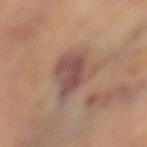Findings:
• notes · no biopsy performed (imaged during a skin exam)
• TBP lesion metrics · an area of roughly 15 mm², a shape eccentricity near 0.65, and two-axis asymmetry of about 0.35; a lesion-detection confidence of about 95/100
• subject · female, aged around 60
• image · ~15 mm crop, total-body skin-cancer survey
• diameter · ≈5.5 mm
• body site · the leg
• tile lighting · cross-polarized illumination